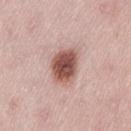Recorded during total-body skin imaging; not selected for excision or biopsy. A lesion tile, about 15 mm wide, cut from a 3D total-body photograph. On the back. A female patient aged 38 to 42. The tile uses white-light illumination. The lesion's longest dimension is about 4.5 mm.